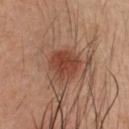• notes · catalogued during a skin exam; not biopsied
• image source · 15 mm crop, total-body photography
• subject · male, aged 48–52
• anatomic site · the head or neck